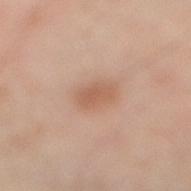Findings:
* notes · catalogued during a skin exam; not biopsied
* tile lighting · cross-polarized illumination
* image · 15 mm crop, total-body photography
* site · the right lower leg
* patient · male, in their mid-50s